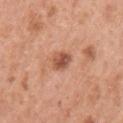Captured during whole-body skin photography for melanoma surveillance; the lesion was not biopsied. The lesion's longest dimension is about 3 mm. Automated tile analysis of the lesion measured a footprint of about 5 mm², an eccentricity of roughly 0.7, and a symmetry-axis asymmetry near 0.2. And it measured about 12 CIELAB-L* units darker than the surrounding skin and a lesion-to-skin contrast of about 8 (normalized; higher = more distinct). The analysis additionally found a nevus-likeness score of about 70/100 and a lesion-detection confidence of about 100/100. This is a white-light tile. A region of skin cropped from a whole-body photographic capture, roughly 15 mm wide. Located on the left upper arm. The subject is a female aged approximately 50.